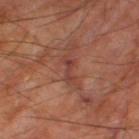Notes:
- workup — total-body-photography surveillance lesion; no biopsy
- subject — male, about 70 years old
- acquisition — total-body-photography crop, ~15 mm field of view
- site — the leg
- lesion diameter — about 3 mm